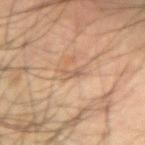| feature | finding |
|---|---|
| follow-up | imaged on a skin check; not biopsied |
| imaging modality | total-body-photography crop, ~15 mm field of view |
| location | the left forearm |
| automated metrics | an eccentricity of roughly 0.95; border irregularity of about 6 on a 0–10 scale and peripheral color asymmetry of about 0 |
| size | ≈2.5 mm |
| patient | male, aged 58–62 |